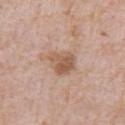Notes:
- workup: imaged on a skin check; not biopsied
- subject: male, aged 48–52
- imaging modality: ~15 mm tile from a whole-body skin photo
- location: the right upper arm
- size: ~3.5 mm (longest diameter)
- lighting: white-light